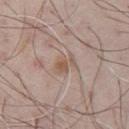The lesion was photographed on a routine skin check and not biopsied; there is no pathology result. An algorithmic analysis of the crop reported a border-irregularity index near 4/10 and internal color variation of about 2 on a 0–10 scale. It also reported lesion-presence confidence of about 100/100. Imaged with white-light lighting. A male patient, roughly 70 years of age. From the chest. A lesion tile, about 15 mm wide, cut from a 3D total-body photograph.